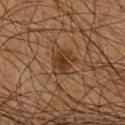Part of a total-body skin-imaging series; this lesion was reviewed on a skin check and was not flagged for biopsy. The subject is a male approximately 65 years of age. Automated image analysis of the tile measured an eccentricity of roughly 0.55 and two-axis asymmetry of about 0.25. It also reported an average lesion color of about L≈30 a*≈17 b*≈27 (CIELAB) and roughly 8 lightness units darker than nearby skin. A close-up tile cropped from a whole-body skin photograph, about 15 mm across. Measured at roughly 3.5 mm in maximum diameter. From the chest.